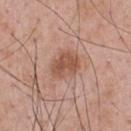Impression: Recorded during total-body skin imaging; not selected for excision or biopsy. Background: From the upper back. Automated image analysis of the tile measured a lesion area of about 9.5 mm², an eccentricity of roughly 0.6, and a symmetry-axis asymmetry near 0.2. It also reported radial color variation of about 1. This image is a 15 mm lesion crop taken from a total-body photograph. A male patient approximately 55 years of age. Longest diameter approximately 4 mm.